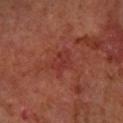Captured during whole-body skin photography for melanoma surveillance; the lesion was not biopsied. This is a cross-polarized tile. The lesion-visualizer software estimated a lesion area of about 5 mm². It also reported a lesion color around L≈34 a*≈29 b*≈27 in CIELAB, roughly 5 lightness units darker than nearby skin, and a normalized border contrast of about 5. And it measured a border-irregularity rating of about 4/10, a within-lesion color-variation index near 1.5/10, and a peripheral color-asymmetry measure near 0.5. And it measured an automated nevus-likeness rating near 0 out of 100 and a lesion-detection confidence of about 100/100. A 15 mm close-up tile from a total-body photography series done for melanoma screening. A male patient in their 60s. The lesion's longest dimension is about 3 mm. The lesion is located on the right forearm.